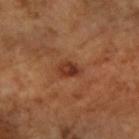Recorded during total-body skin imaging; not selected for excision or biopsy.
Approximately 2.5 mm at its widest.
From the right forearm.
A lesion tile, about 15 mm wide, cut from a 3D total-body photograph.
Captured under cross-polarized illumination.
The subject is a female aged 68 to 72.